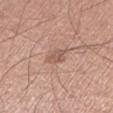The lesion was photographed on a routine skin check and not biopsied; there is no pathology result.
A 15 mm crop from a total-body photograph taken for skin-cancer surveillance.
Located on the left lower leg.
A male patient, in their 60s.